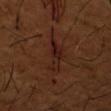The tile uses cross-polarized illumination.
Longest diameter approximately 5 mm.
A male subject, about 65 years old.
Located on the left forearm.
Cropped from a total-body skin-imaging series; the visible field is about 15 mm.
The lesion-visualizer software estimated a lesion area of about 7 mm², a shape eccentricity near 0.95, and a symmetry-axis asymmetry near 0.45. It also reported roughly 6 lightness units darker than nearby skin and a normalized border contrast of about 7.5. It also reported border irregularity of about 5.5 on a 0–10 scale and radial color variation of about 1. And it measured an automated nevus-likeness rating near 20 out of 100 and a lesion-detection confidence of about 80/100.